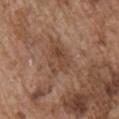Part of a total-body skin-imaging series; this lesion was reviewed on a skin check and was not flagged for biopsy.
A male patient, aged approximately 75.
Located on the right upper arm.
Cropped from a total-body skin-imaging series; the visible field is about 15 mm.
Imaged with white-light lighting.
The recorded lesion diameter is about 4 mm.